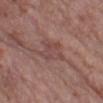Impression:
Recorded during total-body skin imaging; not selected for excision or biopsy.
Context:
The recorded lesion diameter is about 4.5 mm. A region of skin cropped from a whole-body photographic capture, roughly 15 mm wide. A female patient aged approximately 85 or older. On the left lower leg.